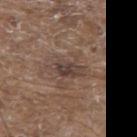Q: Is there a histopathology result?
A: no biopsy performed (imaged during a skin exam)
Q: What is the lesion's diameter?
A: about 4 mm
Q: Automated lesion metrics?
A: an average lesion color of about L≈41 a*≈14 b*≈22 (CIELAB); a within-lesion color-variation index near 4.5/10 and peripheral color asymmetry of about 1.5; a nevus-likeness score of about 0/100
Q: How was the tile lit?
A: white-light
Q: How was this image acquired?
A: ~15 mm tile from a whole-body skin photo
Q: Patient demographics?
A: male, aged approximately 80
Q: Where on the body is the lesion?
A: the upper back